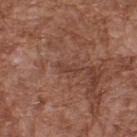| field | value |
|---|---|
| automated lesion analysis | a lesion color around L≈41 a*≈21 b*≈26 in CIELAB and a normalized border contrast of about 5.5; a border-irregularity rating of about 3.5/10, a color-variation rating of about 0/10, and peripheral color asymmetry of about 0; a nevus-likeness score of about 0/100 and a lesion-detection confidence of about 75/100 |
| patient | male, aged 73 to 77 |
| acquisition | ~15 mm crop, total-body skin-cancer survey |
| tile lighting | white-light illumination |
| location | the upper back |